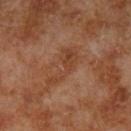{
  "biopsy_status": "not biopsied; imaged during a skin examination",
  "lesion_size": {
    "long_diameter_mm_approx": 5.5
  },
  "site": "leg",
  "image": {
    "source": "total-body photography crop",
    "field_of_view_mm": 15
  },
  "patient": {
    "sex": "male",
    "age_approx": 70
  }
}